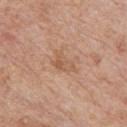Impression: The lesion was photographed on a routine skin check and not biopsied; there is no pathology result. Acquisition and patient details: A male subject about 60 years old. The lesion is on the chest. This image is a 15 mm lesion crop taken from a total-body photograph. Captured under white-light illumination.